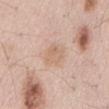Notes:
* follow-up — total-body-photography surveillance lesion; no biopsy
* patient — male, aged approximately 55
* acquisition — ~15 mm tile from a whole-body skin photo
* body site — the mid back
* tile lighting — white-light illumination
* lesion size — ≈3.5 mm
* TBP lesion metrics — a footprint of about 7 mm², a shape eccentricity near 0.7, and two-axis asymmetry of about 0.25